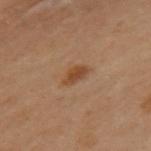This lesion was catalogued during total-body skin photography and was not selected for biopsy.